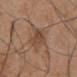{
  "biopsy_status": "not biopsied; imaged during a skin examination",
  "site": "mid back",
  "lighting": "white-light",
  "image": {
    "source": "total-body photography crop",
    "field_of_view_mm": 15
  },
  "lesion_size": {
    "long_diameter_mm_approx": 4.0
  },
  "patient": {
    "sex": "male",
    "age_approx": 55
  }
}